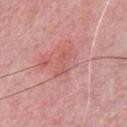No biopsy was performed on this lesion — it was imaged during a full skin examination and was not determined to be concerning.
A male patient aged approximately 50.
A 15 mm crop from a total-body photograph taken for skin-cancer surveillance.
Automated image analysis of the tile measured border irregularity of about 5 on a 0–10 scale and peripheral color asymmetry of about 0.5. The analysis additionally found an automated nevus-likeness rating near 0 out of 100 and a lesion-detection confidence of about 85/100.
The recorded lesion diameter is about 3.5 mm.
The lesion is located on the chest.